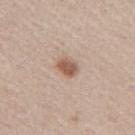| feature | finding |
|---|---|
| imaging modality | 15 mm crop, total-body photography |
| size | ~2.5 mm (longest diameter) |
| location | the arm |
| automated metrics | a lesion area of about 4.5 mm², a shape eccentricity near 0.75, and a symmetry-axis asymmetry near 0.25; an average lesion color of about L≈56 a*≈18 b*≈28 (CIELAB), about 13 CIELAB-L* units darker than the surrounding skin, and a normalized lesion–skin contrast near 8.5; border irregularity of about 2 on a 0–10 scale, a color-variation rating of about 2.5/10, and peripheral color asymmetry of about 1; an automated nevus-likeness rating near 95 out of 100 and lesion-presence confidence of about 100/100 |
| patient | female, in their 60s |